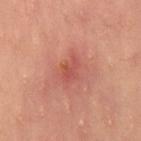follow-up — no biopsy performed (imaged during a skin exam)
patient — female, aged around 35
lighting — cross-polarized
image source — total-body-photography crop, ~15 mm field of view
lesion size — about 2.5 mm
location — the right thigh